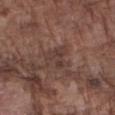Assessment: Recorded during total-body skin imaging; not selected for excision or biopsy. Acquisition and patient details: The lesion is located on the right forearm. A lesion tile, about 15 mm wide, cut from a 3D total-body photograph. The patient is a male approximately 75 years of age. Longest diameter approximately 3 mm. This is a white-light tile. The total-body-photography lesion software estimated a lesion color around L≈38 a*≈17 b*≈22 in CIELAB, about 6 CIELAB-L* units darker than the surrounding skin, and a normalized lesion–skin contrast near 5.5. And it measured a color-variation rating of about 3/10 and radial color variation of about 1.